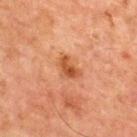Q: Was a biopsy performed?
A: total-body-photography surveillance lesion; no biopsy
Q: Illumination type?
A: cross-polarized
Q: Patient demographics?
A: male, in their mid-60s
Q: What is the anatomic site?
A: the front of the torso
Q: Automated lesion metrics?
A: a footprint of about 4.5 mm² and a shape eccentricity near 0.75; border irregularity of about 4 on a 0–10 scale and radial color variation of about 1; a classifier nevus-likeness of about 80/100 and lesion-presence confidence of about 100/100
Q: What kind of image is this?
A: ~15 mm crop, total-body skin-cancer survey